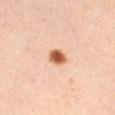Case summary:
- workup — no biopsy performed (imaged during a skin exam)
- tile lighting — cross-polarized
- imaging modality — ~15 mm tile from a whole-body skin photo
- patient — female, aged 38–42
- size — about 2.5 mm
- location — the left thigh